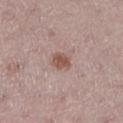- workup — catalogued during a skin exam; not biopsied
- acquisition — 15 mm crop, total-body photography
- subject — female, roughly 45 years of age
- image-analysis metrics — a border-irregularity rating of about 1.5/10 and a color-variation rating of about 2.5/10
- body site — the left lower leg
- lesion diameter — about 2.5 mm
- tile lighting — white-light